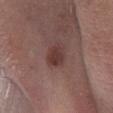imaging modality: ~15 mm tile from a whole-body skin photo | illumination: white-light illumination | automated metrics: an average lesion color of about L≈37 a*≈20 b*≈20 (CIELAB) and a normalized lesion–skin contrast near 8; a border-irregularity rating of about 1.5/10 and peripheral color asymmetry of about 1 | diameter: ≈3 mm | body site: the left lower leg | patient: male, aged approximately 70.